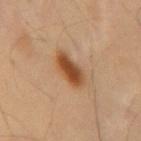{"biopsy_status": "not biopsied; imaged during a skin examination", "image": {"source": "total-body photography crop", "field_of_view_mm": 15}, "automated_metrics": {"nevus_likeness_0_100": 100, "lesion_detection_confidence_0_100": 100}, "lesion_size": {"long_diameter_mm_approx": 4.5}, "patient": {"sex": "male", "age_approx": 55}, "site": "back", "lighting": "cross-polarized"}